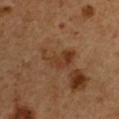Q: Where on the body is the lesion?
A: the right upper arm
Q: What kind of image is this?
A: ~15 mm crop, total-body skin-cancer survey
Q: What are the patient's age and sex?
A: male, aged 48–52
Q: What is the lesion's diameter?
A: ≈4.5 mm
Q: What did automated image analysis measure?
A: a footprint of about 7.5 mm² and two-axis asymmetry of about 0.55; a lesion color around L≈37 a*≈20 b*≈32 in CIELAB and roughly 8 lightness units darker than nearby skin